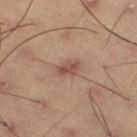Q: Was this lesion biopsied?
A: total-body-photography surveillance lesion; no biopsy
Q: What lighting was used for the tile?
A: cross-polarized
Q: Automated lesion metrics?
A: a mean CIELAB color near L≈42 a*≈17 b*≈22, a lesion–skin lightness drop of about 8, and a lesion-to-skin contrast of about 7 (normalized; higher = more distinct); border irregularity of about 2.5 on a 0–10 scale, a within-lesion color-variation index near 3/10, and peripheral color asymmetry of about 1; a classifier nevus-likeness of about 10/100 and lesion-presence confidence of about 100/100
Q: Lesion location?
A: the left thigh
Q: What is the imaging modality?
A: ~15 mm tile from a whole-body skin photo
Q: Who is the patient?
A: male, aged approximately 55
Q: Lesion size?
A: ~3 mm (longest diameter)